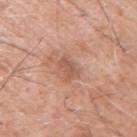Recorded during total-body skin imaging; not selected for excision or biopsy. The lesion is located on the right upper arm. The subject is a male approximately 60 years of age. A close-up tile cropped from a whole-body skin photograph, about 15 mm across.